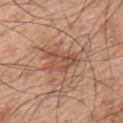This lesion was catalogued during total-body skin photography and was not selected for biopsy. On the upper back. A lesion tile, about 15 mm wide, cut from a 3D total-body photograph. A male subject aged approximately 50. Measured at roughly 4.5 mm in maximum diameter.